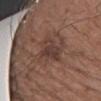follow-up: no biopsy performed (imaged during a skin exam)
body site: the arm
illumination: white-light illumination
subject: male, aged 53–57
acquisition: 15 mm crop, total-body photography
TBP lesion metrics: an area of roughly 8.5 mm² and a shape eccentricity near 0.85; internal color variation of about 4 on a 0–10 scale; a nevus-likeness score of about 10/100 and a detector confidence of about 100 out of 100 that the crop contains a lesion
lesion diameter: ≈4.5 mm